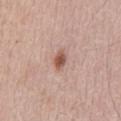Clinical impression: The lesion was tiled from a total-body skin photograph and was not biopsied.